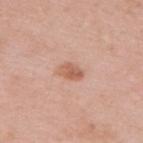The lesion was tiled from a total-body skin photograph and was not biopsied. A female patient, aged 38 to 42. Automated image analysis of the tile measured a lesion area of about 4 mm² and an outline eccentricity of about 0.75 (0 = round, 1 = elongated). The software also gave an average lesion color of about L≈60 a*≈24 b*≈31 (CIELAB). Cropped from a total-body skin-imaging series; the visible field is about 15 mm. Imaged with white-light lighting. The lesion is located on the upper back. The lesion's longest dimension is about 3 mm.